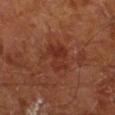Case summary:
- acquisition: total-body-photography crop, ~15 mm field of view
- patient: male, in their mid- to late 60s
- body site: the right lower leg